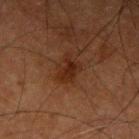The lesion was tiled from a total-body skin photograph and was not biopsied. This image is a 15 mm lesion crop taken from a total-body photograph. On the right upper arm. A male subject aged 73–77.